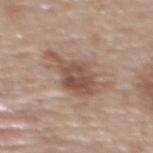Impression: Captured during whole-body skin photography for melanoma surveillance; the lesion was not biopsied. Context: The patient is a female approximately 60 years of age. Imaged with white-light lighting. Cropped from a whole-body photographic skin survey; the tile spans about 15 mm. Longest diameter approximately 6.5 mm. From the back.